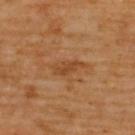The lesion was tiled from a total-body skin photograph and was not biopsied.
The tile uses cross-polarized illumination.
A 15 mm close-up extracted from a 3D total-body photography capture.
From the back.
A male subject aged 58–62.
Longest diameter approximately 3.5 mm.